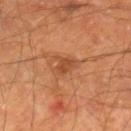<case>
<biopsy_status>not biopsied; imaged during a skin examination</biopsy_status>
<patient>
  <sex>male</sex>
  <age_approx>65</age_approx>
</patient>
<image>
  <source>total-body photography crop</source>
  <field_of_view_mm>15</field_of_view_mm>
</image>
<site>right lower leg</site>
</case>